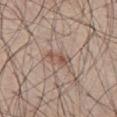No biopsy was performed on this lesion — it was imaged during a full skin examination and was not determined to be concerning.
The lesion is located on the front of the torso.
Cropped from a total-body skin-imaging series; the visible field is about 15 mm.
A male subject, aged approximately 50.